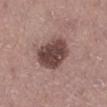acquisition: ~15 mm crop, total-body skin-cancer survey | automated metrics: an area of roughly 15 mm² and a shape eccentricity near 0.65; an average lesion color of about L≈43 a*≈18 b*≈20 (CIELAB), a lesion–skin lightness drop of about 16, and a lesion-to-skin contrast of about 11.5 (normalized; higher = more distinct); a nevus-likeness score of about 35/100 and lesion-presence confidence of about 100/100 | subject: female, aged 48 to 52 | body site: the right lower leg.